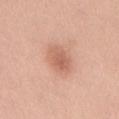follow-up: imaged on a skin check; not biopsied
automated metrics: an area of roughly 9.5 mm², a shape eccentricity near 0.75, and a shape-asymmetry score of about 0.15 (0 = symmetric); an average lesion color of about L≈62 a*≈23 b*≈31 (CIELAB), about 9 CIELAB-L* units darker than the surrounding skin, and a normalized border contrast of about 6; a border-irregularity rating of about 1.5/10
patient: male, aged approximately 40
diameter: ≈4 mm
tile lighting: white-light illumination
site: the abdomen
acquisition: 15 mm crop, total-body photography